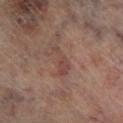notes: total-body-photography surveillance lesion; no biopsy | imaging modality: 15 mm crop, total-body photography | patient: male, aged around 65 | location: the right lower leg.